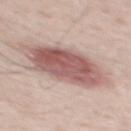The total-body-photography lesion software estimated a shape eccentricity near 0.85 and two-axis asymmetry of about 0.15. The software also gave a border-irregularity index near 2/10, a color-variation rating of about 5.5/10, and a peripheral color-asymmetry measure near 1.5. It also reported a nevus-likeness score of about 95/100 and a detector confidence of about 100 out of 100 that the crop contains a lesion. A close-up tile cropped from a whole-body skin photograph, about 15 mm across. On the mid back. The recorded lesion diameter is about 9 mm. A male patient aged 38 to 42.